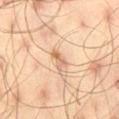Recorded during total-body skin imaging; not selected for excision or biopsy. The tile uses cross-polarized illumination. A male subject, about 45 years old. Measured at roughly 2.5 mm in maximum diameter. Cropped from a whole-body photographic skin survey; the tile spans about 15 mm. On the right thigh.